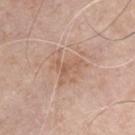biopsy status — imaged on a skin check; not biopsied | subject — male, in their mid- to late 60s | size — ≈3 mm | image source — 15 mm crop, total-body photography | anatomic site — the chest | lighting — white-light.